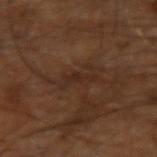| key | value |
|---|---|
| follow-up | total-body-photography surveillance lesion; no biopsy |
| image-analysis metrics | a lesion area of about 3 mm², an outline eccentricity of about 0.9 (0 = round, 1 = elongated), and two-axis asymmetry of about 0.45; a border-irregularity rating of about 5.5/10 and a color-variation rating of about 0/10 |
| body site | the arm |
| imaging modality | total-body-photography crop, ~15 mm field of view |
| patient | male, aged approximately 60 |